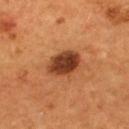Impression: Part of a total-body skin-imaging series; this lesion was reviewed on a skin check and was not flagged for biopsy. Context: A close-up tile cropped from a whole-body skin photograph, about 15 mm across. The lesion-visualizer software estimated an automated nevus-likeness rating near 90 out of 100. Located on the upper back. A male subject, aged 53–57.